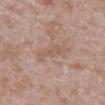biopsy_status: not biopsied; imaged during a skin examination
patient:
  sex: male
  age_approx: 60
lesion_size:
  long_diameter_mm_approx: 4.5
site: right lower leg
automated_metrics:
  cielab_L: 57
  cielab_a: 18
  cielab_b: 26
  vs_skin_darker_L: 6.0
  vs_skin_contrast_norm: 5.0
  border_irregularity_0_10: 6.0
  color_variation_0_10: 1.5
  peripheral_color_asymmetry: 0.5
  nevus_likeness_0_100: 0
image:
  source: total-body photography crop
  field_of_view_mm: 15
lighting: white-light